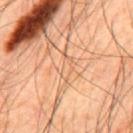Impression:
No biopsy was performed on this lesion — it was imaged during a full skin examination and was not determined to be concerning.
Image and clinical context:
A region of skin cropped from a whole-body photographic capture, roughly 15 mm wide. A male patient aged 43 to 47. The lesion is on the mid back. The total-body-photography lesion software estimated an average lesion color of about L≈63 a*≈21 b*≈36 (CIELAB), roughly 5 lightness units darker than nearby skin, and a normalized border contrast of about 3.5. The software also gave a nevus-likeness score of about 0/100.